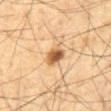Assessment: Captured during whole-body skin photography for melanoma surveillance; the lesion was not biopsied. Background: Located on the abdomen. Cropped from a total-body skin-imaging series; the visible field is about 15 mm. This is a cross-polarized tile. Longest diameter approximately 2.5 mm. A male subject in their mid-60s. An algorithmic analysis of the crop reported an outline eccentricity of about 0.5 (0 = round, 1 = elongated). And it measured a border-irregularity index near 2/10, a within-lesion color-variation index near 6/10, and peripheral color asymmetry of about 2.